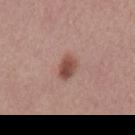Assessment:
The lesion was tiled from a total-body skin photograph and was not biopsied.
Clinical summary:
A male subject, aged 58 to 62. Automated image analysis of the tile measured an area of roughly 5 mm², an outline eccentricity of about 0.75 (0 = round, 1 = elongated), and two-axis asymmetry of about 0.25. And it measured about 13 CIELAB-L* units darker than the surrounding skin. The software also gave border irregularity of about 2 on a 0–10 scale, a within-lesion color-variation index near 4/10, and radial color variation of about 1.5. The analysis additionally found an automated nevus-likeness rating near 95 out of 100 and a detector confidence of about 100 out of 100 that the crop contains a lesion. The tile uses white-light illumination. The lesion is located on the mid back. The lesion's longest dimension is about 3 mm. This image is a 15 mm lesion crop taken from a total-body photograph.